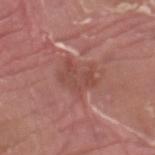{"biopsy_status": "not biopsied; imaged during a skin examination"}